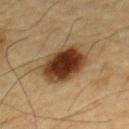The lesion was photographed on a routine skin check and not biopsied; there is no pathology result.
An algorithmic analysis of the crop reported a classifier nevus-likeness of about 100/100 and lesion-presence confidence of about 100/100.
Imaged with cross-polarized lighting.
A region of skin cropped from a whole-body photographic capture, roughly 15 mm wide.
Located on the chest.
Approximately 5 mm at its widest.
The patient is a male approximately 85 years of age or older.